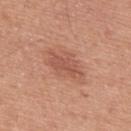Part of a total-body skin-imaging series; this lesion was reviewed on a skin check and was not flagged for biopsy. From the upper back. A male subject in their mid- to late 50s. Cropped from a total-body skin-imaging series; the visible field is about 15 mm. Longest diameter approximately 5 mm. Automated tile analysis of the lesion measured an area of roughly 12 mm², an outline eccentricity of about 0.8 (0 = round, 1 = elongated), and a symmetry-axis asymmetry near 0.2. The software also gave a lesion color around L≈55 a*≈24 b*≈30 in CIELAB, a lesion–skin lightness drop of about 8, and a lesion-to-skin contrast of about 5.5 (normalized; higher = more distinct). The analysis additionally found border irregularity of about 3 on a 0–10 scale and internal color variation of about 3 on a 0–10 scale. The software also gave a nevus-likeness score of about 30/100. The tile uses white-light illumination.